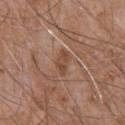Context: A region of skin cropped from a whole-body photographic capture, roughly 15 mm wide. The lesion is on the upper back. A male patient, roughly 60 years of age. Longest diameter approximately 3 mm.